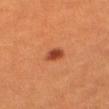Findings:
• biopsy status — no biopsy performed (imaged during a skin exam)
• subject — female, approximately 30 years of age
• lesion size — about 3 mm
• tile lighting — cross-polarized illumination
• imaging modality — total-body-photography crop, ~15 mm field of view
• site — the right thigh